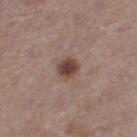biopsy status: imaged on a skin check; not biopsied
size: ~2.5 mm (longest diameter)
image source: 15 mm crop, total-body photography
subject: female, aged 58–62
illumination: white-light illumination
body site: the left thigh